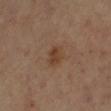Recorded during total-body skin imaging; not selected for excision or biopsy.
A close-up tile cropped from a whole-body skin photograph, about 15 mm across.
Located on the left lower leg.
A female subject, approximately 70 years of age.